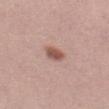Clinical summary:
The lesion-visualizer software estimated an area of roughly 4 mm², an outline eccentricity of about 0.75 (0 = round, 1 = elongated), and a shape-asymmetry score of about 0.2 (0 = symmetric). It also reported a normalized lesion–skin contrast near 9. The analysis additionally found a border-irregularity rating of about 1.5/10 and radial color variation of about 0.5. A 15 mm close-up extracted from a 3D total-body photography capture. Measured at roughly 2.5 mm in maximum diameter. The patient is a female approximately 20 years of age. Imaged with white-light lighting. On the left thigh.